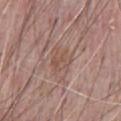Findings:
• follow-up: total-body-photography surveillance lesion; no biopsy
• body site: the chest
• image: ~15 mm crop, total-body skin-cancer survey
• subject: male, about 70 years old
• lighting: white-light